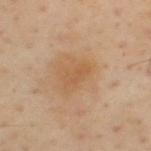The lesion was photographed on a routine skin check and not biopsied; there is no pathology result.
The recorded lesion diameter is about 3 mm.
Captured under cross-polarized illumination.
From the upper back.
A male patient, in their mid-50s.
Automated tile analysis of the lesion measured an area of roughly 4.5 mm² and an eccentricity of roughly 0.85. And it measured an average lesion color of about L≈56 a*≈20 b*≈37 (CIELAB), a lesion–skin lightness drop of about 6, and a normalized border contrast of about 4.5. And it measured a detector confidence of about 100 out of 100 that the crop contains a lesion.
This image is a 15 mm lesion crop taken from a total-body photograph.